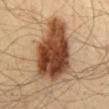  biopsy_status: not biopsied; imaged during a skin examination
  image:
    source: total-body photography crop
    field_of_view_mm: 15
  site: mid back
  patient:
    sex: male
    age_approx: 35
  lighting: cross-polarized
  automated_metrics:
    area_mm2_approx: 37.0
    cielab_L: 42
    cielab_a: 19
    cielab_b: 30
    vs_skin_darker_L: 18.0
    vs_skin_contrast_norm: 13.5
    border_irregularity_0_10: 3.5
    color_variation_0_10: 7.5
    peripheral_color_asymmetry: 2.5
  lesion_size:
    long_diameter_mm_approx: 8.5